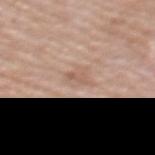This lesion was catalogued during total-body skin photography and was not selected for biopsy. A female subject, aged 53 to 57. Cropped from a total-body skin-imaging series; the visible field is about 15 mm. The lesion is located on the upper back.